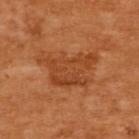Q: Was this lesion biopsied?
A: catalogued during a skin exam; not biopsied